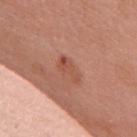Q: What is the lesion's diameter?
A: ≈3.5 mm
Q: Patient demographics?
A: female, aged 58 to 62
Q: What kind of image is this?
A: ~15 mm tile from a whole-body skin photo
Q: What lighting was used for the tile?
A: white-light
Q: Where on the body is the lesion?
A: the upper back